A close-up tile cropped from a whole-body skin photograph, about 15 mm across; a male subject aged approximately 70; from the upper back:
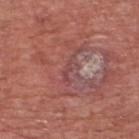Q: How was the tile lit?
A: white-light illumination
Q: How large is the lesion?
A: ≈3 mm
Q: What did automated image analysis measure?
A: a footprint of about 3 mm² and a shape eccentricity near 0.9; a lesion–skin lightness drop of about 6 and a normalized border contrast of about 5
Q: What did the biopsy show?
A: a seborrheic keratosis